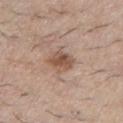Findings:
- workup — total-body-photography surveillance lesion; no biopsy
- location — the chest
- subject — male, in their mid- to late 60s
- imaging modality — ~15 mm crop, total-body skin-cancer survey